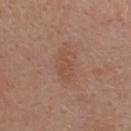notes = total-body-photography surveillance lesion; no biopsy | tile lighting = white-light illumination | image source = ~15 mm crop, total-body skin-cancer survey | subject = female, aged around 30 | lesion diameter = ~4 mm (longest diameter) | automated lesion analysis = a lesion area of about 7.5 mm², an eccentricity of roughly 0.8, and two-axis asymmetry of about 0.4; a lesion color around L≈50 a*≈19 b*≈29 in CIELAB, a lesion–skin lightness drop of about 5, and a normalized lesion–skin contrast near 4.5; a nevus-likeness score of about 0/100 | location = the back.